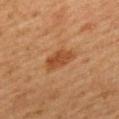* notes · no biopsy performed (imaged during a skin exam)
* patient · female, in their 50s
* illumination · cross-polarized illumination
* location · the back
* image · 15 mm crop, total-body photography